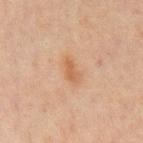Impression: Recorded during total-body skin imaging; not selected for excision or biopsy. Context: Automated tile analysis of the lesion measured a border-irregularity index near 3/10 and internal color variation of about 1 on a 0–10 scale. A roughly 15 mm field-of-view crop from a total-body skin photograph. Imaged with cross-polarized lighting. On the mid back. A male patient aged approximately 70.